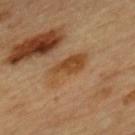Assessment: Recorded during total-body skin imaging; not selected for excision or biopsy. Context: The patient is a female roughly 60 years of age. Cropped from a whole-body photographic skin survey; the tile spans about 15 mm. Located on the mid back.